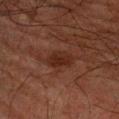notes = no biopsy performed (imaged during a skin exam); acquisition = 15 mm crop, total-body photography; patient = male, aged approximately 80; site = the arm; tile lighting = cross-polarized illumination.